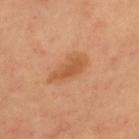<case>
  <biopsy_status>not biopsied; imaged during a skin examination</biopsy_status>
  <lesion_size>
    <long_diameter_mm_approx>5.0</long_diameter_mm_approx>
  </lesion_size>
  <automated_metrics>
    <cielab_L>55</cielab_L>
    <cielab_a>24</cielab_a>
    <cielab_b>39</cielab_b>
    <vs_skin_darker_L>8.0</vs_skin_darker_L>
    <vs_skin_contrast_norm>6.5</vs_skin_contrast_norm>
  </automated_metrics>
  <lighting>cross-polarized</lighting>
  <image>
    <source>total-body photography crop</source>
    <field_of_view_mm>15</field_of_view_mm>
  </image>
  <patient>
    <sex>male</sex>
    <age_approx>55</age_approx>
  </patient>
  <site>upper back</site>
</case>